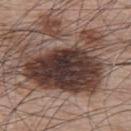notes — catalogued during a skin exam; not biopsied
size — ≈12 mm
body site — the upper back
imaging modality — ~15 mm crop, total-body skin-cancer survey
subject — male, aged around 65